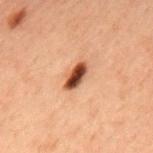notes: no biopsy performed (imaged during a skin exam)
location: the upper back
image source: ~15 mm tile from a whole-body skin photo
diameter: about 3.5 mm
automated lesion analysis: a lesion-to-skin contrast of about 14 (normalized; higher = more distinct); a color-variation rating of about 4.5/10 and a peripheral color-asymmetry measure near 1.5; a nevus-likeness score of about 100/100 and a lesion-detection confidence of about 100/100
patient: male, in their 60s